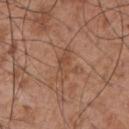Clinical impression: Imaged during a routine full-body skin examination; the lesion was not biopsied and no histopathology is available. Image and clinical context: The total-body-photography lesion software estimated an outline eccentricity of about 0.9 (0 = round, 1 = elongated). The analysis additionally found a lesion color around L≈48 a*≈21 b*≈30 in CIELAB, about 7 CIELAB-L* units darker than the surrounding skin, and a lesion-to-skin contrast of about 5.5 (normalized; higher = more distinct). It also reported internal color variation of about 3 on a 0–10 scale and peripheral color asymmetry of about 0.5. The patient is a male aged approximately 55. Located on the chest. Imaged with white-light lighting. About 4 mm across. Cropped from a total-body skin-imaging series; the visible field is about 15 mm.